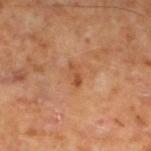The lesion was photographed on a routine skin check and not biopsied; there is no pathology result.